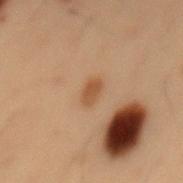Findings:
– follow-up · catalogued during a skin exam; not biopsied
– body site · the mid back
– automated lesion analysis · a lesion area of about 3 mm², an outline eccentricity of about 0.85 (0 = round, 1 = elongated), and two-axis asymmetry of about 0.15; a nevus-likeness score of about 0/100 and a detector confidence of about 100 out of 100 that the crop contains a lesion
– patient · male, roughly 55 years of age
– lighting · cross-polarized
– imaging modality · total-body-photography crop, ~15 mm field of view
– size · ≈2.5 mm